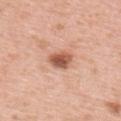Findings:
– biopsy status · total-body-photography surveillance lesion; no biopsy
– body site · the upper back
– image · ~15 mm tile from a whole-body skin photo
– lighting · white-light illumination
– image-analysis metrics · an area of roughly 6 mm², an eccentricity of roughly 0.75, and a symmetry-axis asymmetry near 0.2; a border-irregularity rating of about 2/10, a within-lesion color-variation index near 4.5/10, and a peripheral color-asymmetry measure near 1.5; a nevus-likeness score of about 90/100 and a lesion-detection confidence of about 100/100
– subject · female, aged 38 to 42